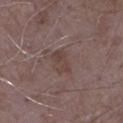Imaged during a routine full-body skin examination; the lesion was not biopsied and no histopathology is available.
The patient is a male aged 63–67.
The lesion is on the left upper arm.
A 15 mm close-up tile from a total-body photography series done for melanoma screening.